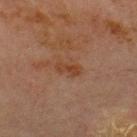A male patient aged around 70. Approximately 3 mm at its widest. Captured under cross-polarized illumination. On the chest. A region of skin cropped from a whole-body photographic capture, roughly 15 mm wide.